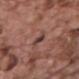{"biopsy_status": "not biopsied; imaged during a skin examination", "image": {"source": "total-body photography crop", "field_of_view_mm": 15}, "patient": {"sex": "male", "age_approx": 70}, "lesion_size": {"long_diameter_mm_approx": 2.5}, "site": "upper back"}